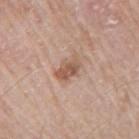biopsy_status: not biopsied; imaged during a skin examination
image:
  source: total-body photography crop
  field_of_view_mm: 15
lesion_size:
  long_diameter_mm_approx: 3.5
lighting: white-light
site: right upper arm
patient:
  sex: male
  age_approx: 65
automated_metrics:
  color_variation_0_10: 3.5